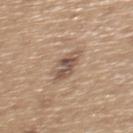{
  "biopsy_status": "not biopsied; imaged during a skin examination",
  "site": "back",
  "image": {
    "source": "total-body photography crop",
    "field_of_view_mm": 15
  },
  "lesion_size": {
    "long_diameter_mm_approx": 3.5
  },
  "patient": {
    "sex": "male",
    "age_approx": 65
  },
  "automated_metrics": {
    "area_mm2_approx": 6.5,
    "eccentricity": 0.8,
    "shape_asymmetry": 0.3
  },
  "lighting": "white-light"
}